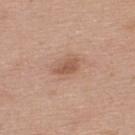Impression: Captured during whole-body skin photography for melanoma surveillance; the lesion was not biopsied. Background: A male patient aged approximately 25. The lesion is located on the upper back. Cropped from a total-body skin-imaging series; the visible field is about 15 mm. Longest diameter approximately 3 mm. The tile uses white-light illumination.